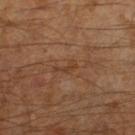<lesion>
  <biopsy_status>not biopsied; imaged during a skin examination</biopsy_status>
  <lighting>cross-polarized</lighting>
  <lesion_size>
    <long_diameter_mm_approx>2.5</long_diameter_mm_approx>
  </lesion_size>
  <automated_metrics>
    <area_mm2_approx>2.0</area_mm2_approx>
    <eccentricity>0.9</eccentricity>
    <shape_asymmetry>0.5</shape_asymmetry>
    <cielab_L>35</cielab_L>
    <cielab_a>18</cielab_a>
    <cielab_b>28</cielab_b>
    <vs_skin_darker_L>6.0</vs_skin_darker_L>
    <vs_skin_contrast_norm>5.5</vs_skin_contrast_norm>
    <border_irregularity_0_10>6.0</border_irregularity_0_10>
    <peripheral_color_asymmetry>0.0</peripheral_color_asymmetry>
    <nevus_likeness_0_100>0</nevus_likeness_0_100>
    <lesion_detection_confidence_0_100>95</lesion_detection_confidence_0_100>
  </automated_metrics>
  <patient>
    <sex>male</sex>
    <age_approx>60</age_approx>
  </patient>
  <site>right lower leg</site>
  <image>
    <source>total-body photography crop</source>
    <field_of_view_mm>15</field_of_view_mm>
  </image>
</lesion>